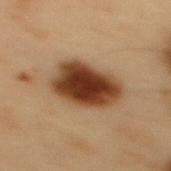follow-up = total-body-photography surveillance lesion; no biopsy
image source = total-body-photography crop, ~15 mm field of view
illumination = cross-polarized illumination
location = the mid back
subject = male, roughly 55 years of age